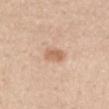No biopsy was performed on this lesion — it was imaged during a full skin examination and was not determined to be concerning.
The recorded lesion diameter is about 3 mm.
The patient is a female aged around 45.
On the back.
The lesion-visualizer software estimated a within-lesion color-variation index near 2/10 and a peripheral color-asymmetry measure near 0.5. It also reported a classifier nevus-likeness of about 45/100 and a lesion-detection confidence of about 100/100.
This image is a 15 mm lesion crop taken from a total-body photograph.